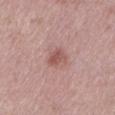Impression:
Captured during whole-body skin photography for melanoma surveillance; the lesion was not biopsied.
Acquisition and patient details:
Longest diameter approximately 2.5 mm. Automated image analysis of the tile measured a mean CIELAB color near L≈53 a*≈23 b*≈24. It also reported an automated nevus-likeness rating near 40 out of 100 and a lesion-detection confidence of about 100/100. Cropped from a whole-body photographic skin survey; the tile spans about 15 mm. The subject is a female about 40 years old. The tile uses white-light illumination. The lesion is located on the left lower leg.